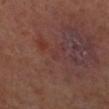Q: Was this lesion biopsied?
A: no biopsy performed (imaged during a skin exam)
Q: What kind of image is this?
A: ~15 mm tile from a whole-body skin photo
Q: How large is the lesion?
A: ~1 mm (longest diameter)
Q: Where on the body is the lesion?
A: the left lower leg
Q: What lighting was used for the tile?
A: cross-polarized
Q: Who is the patient?
A: female